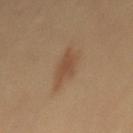Assessment: The lesion was photographed on a routine skin check and not biopsied; there is no pathology result. Clinical summary: This is a cross-polarized tile. Approximately 4 mm at its widest. Cropped from a whole-body photographic skin survey; the tile spans about 15 mm. The patient is a female in their 50s.